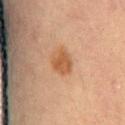follow-up=catalogued during a skin exam; not biopsied
lighting=cross-polarized illumination
patient=male, about 65 years old
body site=the abdomen
image source=~15 mm crop, total-body skin-cancer survey